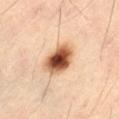Part of a total-body skin-imaging series; this lesion was reviewed on a skin check and was not flagged for biopsy.
Cropped from a total-body skin-imaging series; the visible field is about 15 mm.
The patient is a male aged 53–57.
Approximately 4 mm at its widest.
Captured under cross-polarized illumination.
The lesion is on the left thigh.